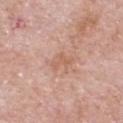workup = no biopsy performed (imaged during a skin exam) | subject = male, aged 53 to 57 | TBP lesion metrics = an automated nevus-likeness rating near 0 out of 100 and lesion-presence confidence of about 100/100 | tile lighting = white-light illumination | size = ≈3.5 mm | site = the chest | image = 15 mm crop, total-body photography.